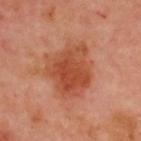Clinical impression: Part of a total-body skin-imaging series; this lesion was reviewed on a skin check and was not flagged for biopsy. Acquisition and patient details: On the upper back. The subject is a male about 50 years old. Automated image analysis of the tile measured an average lesion color of about L≈49 a*≈29 b*≈35 (CIELAB), about 10 CIELAB-L* units darker than the surrounding skin, and a lesion-to-skin contrast of about 8 (normalized; higher = more distinct). The analysis additionally found a border-irregularity rating of about 3.5/10, a color-variation rating of about 5/10, and peripheral color asymmetry of about 1.5. It also reported a detector confidence of about 100 out of 100 that the crop contains a lesion. Cropped from a whole-body photographic skin survey; the tile spans about 15 mm. Approximately 6.5 mm at its widest. Captured under cross-polarized illumination.